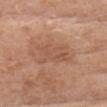The lesion was tiled from a total-body skin photograph and was not biopsied.
This image is a 15 mm lesion crop taken from a total-body photograph.
About 5 mm across.
Captured under white-light illumination.
The lesion-visualizer software estimated a detector confidence of about 100 out of 100 that the crop contains a lesion.
The subject is a female in their 70s.
On the head or neck.